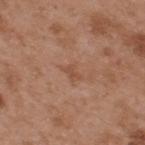Assessment: Recorded during total-body skin imaging; not selected for excision or biopsy. Clinical summary: The lesion-visualizer software estimated a shape eccentricity near 0.8 and a symmetry-axis asymmetry near 0.45. And it measured a mean CIELAB color near L≈50 a*≈22 b*≈31, roughly 6 lightness units darker than nearby skin, and a normalized lesion–skin contrast near 5. The analysis additionally found border irregularity of about 4.5 on a 0–10 scale, a within-lesion color-variation index near 0.5/10, and radial color variation of about 0.5. The software also gave a classifier nevus-likeness of about 0/100. A close-up tile cropped from a whole-body skin photograph, about 15 mm across. Imaged with white-light lighting. The patient is a male in their mid-50s. Measured at roughly 2.5 mm in maximum diameter. The lesion is on the upper back.